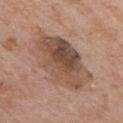| feature | finding |
|---|---|
| biopsy status | no biopsy performed (imaged during a skin exam) |
| image source | ~15 mm tile from a whole-body skin photo |
| illumination | white-light |
| subject | male, approximately 65 years of age |
| site | the chest |
| TBP lesion metrics | an average lesion color of about L≈50 a*≈18 b*≈27 (CIELAB) and a normalized lesion–skin contrast near 8; an automated nevus-likeness rating near 10 out of 100 and lesion-presence confidence of about 100/100 |